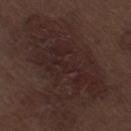Clinical impression: This lesion was catalogued during total-body skin photography and was not selected for biopsy. Image and clinical context: A male subject aged 68 to 72. A region of skin cropped from a whole-body photographic capture, roughly 15 mm wide. About 7.5 mm across. Imaged with white-light lighting. The total-body-photography lesion software estimated a shape eccentricity near 0.9 and a shape-asymmetry score of about 0.65 (0 = symmetric). The analysis additionally found a mean CIELAB color near L≈22 a*≈16 b*≈16, a lesion–skin lightness drop of about 4, and a normalized lesion–skin contrast near 5.5. And it measured a border-irregularity rating of about 10/10, a color-variation rating of about 3/10, and a peripheral color-asymmetry measure near 1. The lesion is on the right thigh.